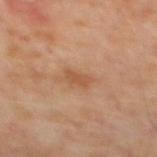<tbp_lesion>
<biopsy_status>not biopsied; imaged during a skin examination</biopsy_status>
<lesion_size>
  <long_diameter_mm_approx>2.5</long_diameter_mm_approx>
</lesion_size>
<lighting>cross-polarized</lighting>
<automated_metrics>
  <area_mm2_approx>3.0</area_mm2_approx>
  <eccentricity>0.8</eccentricity>
  <shape_asymmetry>0.3</shape_asymmetry>
  <border_irregularity_0_10>3.0</border_irregularity_0_10>
  <color_variation_0_10>0.5</color_variation_0_10>
  <peripheral_color_asymmetry>0.0</peripheral_color_asymmetry>
</automated_metrics>
<patient>
  <sex>male</sex>
  <age_approx>70</age_approx>
</patient>
<site>mid back</site>
<image>
  <source>total-body photography crop</source>
  <field_of_view_mm>15</field_of_view_mm>
</image>
</tbp_lesion>